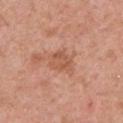Clinical impression: No biopsy was performed on this lesion — it was imaged during a full skin examination and was not determined to be concerning. Background: A female patient, aged approximately 50. The lesion is located on the chest. This image is a 15 mm lesion crop taken from a total-body photograph.